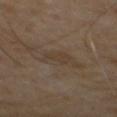Imaged during a routine full-body skin examination; the lesion was not biopsied and no histopathology is available. The patient is a male aged around 65. The tile uses cross-polarized illumination. Cropped from a whole-body photographic skin survey; the tile spans about 15 mm. Measured at roughly 3.5 mm in maximum diameter.